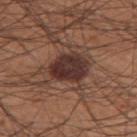<tbp_lesion>
<biopsy_status>not biopsied; imaged during a skin examination</biopsy_status>
</tbp_lesion>